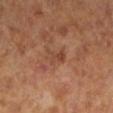Assessment: Imaged during a routine full-body skin examination; the lesion was not biopsied and no histopathology is available. Clinical summary: Automated image analysis of the tile measured an area of roughly 4 mm², a shape eccentricity near 0.75, and a shape-asymmetry score of about 0.25 (0 = symmetric). And it measured a normalized lesion–skin contrast near 5.5. The analysis additionally found lesion-presence confidence of about 100/100. Longest diameter approximately 2.5 mm. The lesion is on the leg. This image is a 15 mm lesion crop taken from a total-body photograph. Captured under cross-polarized illumination. The patient is a female aged approximately 65.